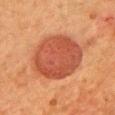  biopsy_status: not biopsied; imaged during a skin examination
  automated_metrics:
    eccentricity: 0.4
    shape_asymmetry: 0.1
    color_variation_0_10: 3.5
  site: chest
  lighting: cross-polarized
  lesion_size:
    long_diameter_mm_approx: 6.5
  image:
    source: total-body photography crop
    field_of_view_mm: 15
  patient:
    sex: female
    age_approx: 50